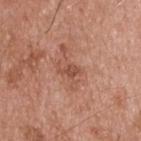Imaged during a routine full-body skin examination; the lesion was not biopsied and no histopathology is available. The lesion is located on the back. A 15 mm close-up tile from a total-body photography series done for melanoma screening. The tile uses white-light illumination. A male subject, aged 53–57.